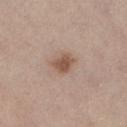  biopsy_status: not biopsied; imaged during a skin examination
  patient:
    sex: female
    age_approx: 65
  lesion_size:
    long_diameter_mm_approx: 2.5
  lighting: white-light
  site: right lower leg
  image:
    source: total-body photography crop
    field_of_view_mm: 15
  automated_metrics:
    cielab_L: 54
    cielab_a: 18
    cielab_b: 28
    vs_skin_darker_L: 11.0
    vs_skin_contrast_norm: 8.0
    nevus_likeness_0_100: 85
    lesion_detection_confidence_0_100: 100